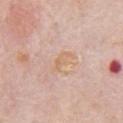{
  "biopsy_status": "not biopsied; imaged during a skin examination",
  "patient": {
    "sex": "male",
    "age_approx": 80
  },
  "image": {
    "source": "total-body photography crop",
    "field_of_view_mm": 15
  },
  "automated_metrics": {
    "area_mm2_approx": 4.0,
    "border_irregularity_0_10": 4.5,
    "color_variation_0_10": 1.0,
    "peripheral_color_asymmetry": 0.0
  },
  "site": "chest",
  "lighting": "white-light"
}